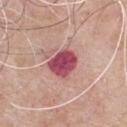Clinical impression: No biopsy was performed on this lesion — it was imaged during a full skin examination and was not determined to be concerning. Context: Captured under white-light illumination. The lesion is on the chest. This image is a 15 mm lesion crop taken from a total-body photograph. The patient is a male in their mid- to late 60s. The lesion's longest dimension is about 3.5 mm.